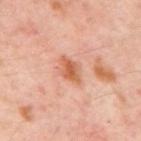No biopsy was performed on this lesion — it was imaged during a full skin examination and was not determined to be concerning.
A lesion tile, about 15 mm wide, cut from a 3D total-body photograph.
Located on the mid back.
This is a cross-polarized tile.
The lesion's longest dimension is about 3.5 mm.
A subject aged 53 to 57.